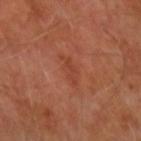Clinical impression: Imaged during a routine full-body skin examination; the lesion was not biopsied and no histopathology is available. Image and clinical context: About 4 mm across. A male subject, aged 48 to 52. Located on the left forearm. The tile uses cross-polarized illumination. A close-up tile cropped from a whole-body skin photograph, about 15 mm across.